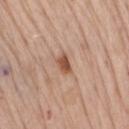This lesion was catalogued during total-body skin photography and was not selected for biopsy. A region of skin cropped from a whole-body photographic capture, roughly 15 mm wide. The lesion is on the back. This is a white-light tile. The patient is a female approximately 75 years of age.